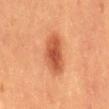follow-up — no biopsy performed (imaged during a skin exam)
automated metrics — a lesion area of about 12 mm², an eccentricity of roughly 0.85, and a shape-asymmetry score of about 0.2 (0 = symmetric); a border-irregularity rating of about 2/10, a color-variation rating of about 3.5/10, and peripheral color asymmetry of about 1; a lesion-detection confidence of about 100/100
imaging modality — ~15 mm tile from a whole-body skin photo
illumination — cross-polarized
site — the back
patient — male, aged approximately 40